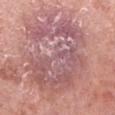<lesion>
<biopsy_status>not biopsied; imaged during a skin examination</biopsy_status>
<lesion_size>
  <long_diameter_mm_approx>11.0</long_diameter_mm_approx>
</lesion_size>
<image>
  <source>total-body photography crop</source>
  <field_of_view_mm>15</field_of_view_mm>
</image>
<patient>
  <sex>male</sex>
  <age_approx>55</age_approx>
</patient>
<site>leg</site>
<lighting>white-light</lighting>
</lesion>